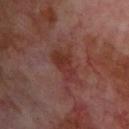lesion_size:
  long_diameter_mm_approx: 4.5
automated_metrics:
  eccentricity: 0.85
  shape_asymmetry: 0.3
  cielab_L: 33
  cielab_a: 24
  cielab_b: 23
  vs_skin_darker_L: 7.0
  vs_skin_contrast_norm: 7.0
  nevus_likeness_0_100: 0
  lesion_detection_confidence_0_100: 100
patient:
  sex: male
  age_approx: 70
lighting: cross-polarized
image:
  source: total-body photography crop
  field_of_view_mm: 15
site: upper back